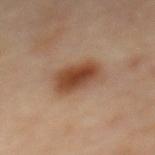biopsy status: catalogued during a skin exam; not biopsied
illumination: cross-polarized illumination
TBP lesion metrics: an average lesion color of about L≈47 a*≈21 b*≈32 (CIELAB), a lesion–skin lightness drop of about 13, and a normalized lesion–skin contrast near 9.5
subject: male, in their mid-50s
image: 15 mm crop, total-body photography
lesion diameter: about 4.5 mm
anatomic site: the mid back